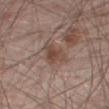Case summary:
* workup: imaged on a skin check; not biopsied
* anatomic site: the right thigh
* image source: ~15 mm crop, total-body skin-cancer survey
* lesion diameter: ~3.5 mm (longest diameter)
* patient: male, aged approximately 65
* image-analysis metrics: a lesion area of about 6.5 mm², an eccentricity of roughly 0.7, and a shape-asymmetry score of about 0.35 (0 = symmetric); a mean CIELAB color near L≈46 a*≈17 b*≈24, roughly 8 lightness units darker than nearby skin, and a lesion-to-skin contrast of about 7 (normalized; higher = more distinct)
* tile lighting: white-light illumination